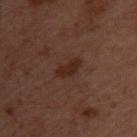Impression:
No biopsy was performed on this lesion — it was imaged during a full skin examination and was not determined to be concerning.
Acquisition and patient details:
From the upper back. A region of skin cropped from a whole-body photographic capture, roughly 15 mm wide. Approximately 3.5 mm at its widest. The tile uses cross-polarized illumination. The subject is a female aged 53–57.